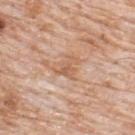{
  "biopsy_status": "not biopsied; imaged during a skin examination",
  "site": "upper back",
  "lighting": "white-light",
  "patient": {
    "sex": "male",
    "age_approx": 80
  },
  "image": {
    "source": "total-body photography crop",
    "field_of_view_mm": 15
  }
}